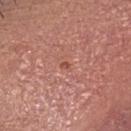Acquisition and patient details:
The tile uses white-light illumination. A female subject, approximately 45 years of age. An algorithmic analysis of the crop reported a shape eccentricity near 0.65 and two-axis asymmetry of about 0.15. The analysis additionally found a lesion color around L≈49 a*≈29 b*≈27 in CIELAB, a lesion–skin lightness drop of about 9, and a normalized lesion–skin contrast near 6.5. A 15 mm close-up tile from a total-body photography series done for melanoma screening. The lesion's longest dimension is about 1 mm. From the head or neck.